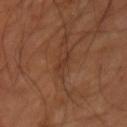Assessment: This lesion was catalogued during total-body skin photography and was not selected for biopsy. Image and clinical context: The recorded lesion diameter is about 3.5 mm. Located on the left upper arm. A male patient aged approximately 50. A 15 mm close-up extracted from a 3D total-body photography capture.